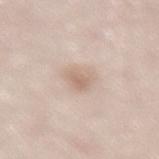| feature | finding |
|---|---|
| notes | no biopsy performed (imaged during a skin exam) |
| patient | female, aged 48 to 52 |
| size | ~2.5 mm (longest diameter) |
| image source | 15 mm crop, total-body photography |
| body site | the lower back |
| tile lighting | white-light |